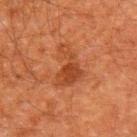Imaged during a routine full-body skin examination; the lesion was not biopsied and no histopathology is available.
On the left upper arm.
Cropped from a whole-body photographic skin survey; the tile spans about 15 mm.
Approximately 4.5 mm at its widest.
Imaged with cross-polarized lighting.
The lesion-visualizer software estimated a mean CIELAB color near L≈35 a*≈24 b*≈32 and a normalized lesion–skin contrast near 7. It also reported a border-irregularity rating of about 6.5/10 and a within-lesion color-variation index near 2.5/10. The analysis additionally found a classifier nevus-likeness of about 10/100 and lesion-presence confidence of about 100/100.
The subject is a male in their 60s.